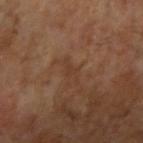No biopsy was performed on this lesion — it was imaged during a full skin examination and was not determined to be concerning. Approximately 3 mm at its widest. A region of skin cropped from a whole-body photographic capture, roughly 15 mm wide. A male patient, aged 63–67. The lesion is located on the right upper arm. An algorithmic analysis of the crop reported internal color variation of about 0 on a 0–10 scale and radial color variation of about 0. Imaged with cross-polarized lighting.